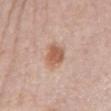Q: Is there a histopathology result?
A: total-body-photography surveillance lesion; no biopsy
Q: Where on the body is the lesion?
A: the mid back
Q: What are the patient's age and sex?
A: male, aged 73 to 77
Q: How was this image acquired?
A: ~15 mm crop, total-body skin-cancer survey